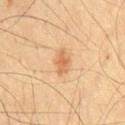notes: catalogued during a skin exam; not biopsied
patient: male, roughly 55 years of age
location: the chest
lesion diameter: ≈3 mm
imaging modality: total-body-photography crop, ~15 mm field of view